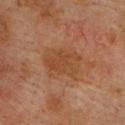follow-up — no biopsy performed (imaged during a skin exam)
anatomic site — the upper back
lighting — cross-polarized illumination
automated lesion analysis — a lesion area of about 13 mm², an outline eccentricity of about 0.7 (0 = round, 1 = elongated), and a shape-asymmetry score of about 0.2 (0 = symmetric); a classifier nevus-likeness of about 0/100
subject — male, aged approximately 75
acquisition — total-body-photography crop, ~15 mm field of view
diameter — ~4.5 mm (longest diameter)